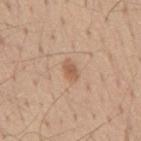Case summary:
- notes · catalogued during a skin exam; not biopsied
- lesion diameter · ~2.5 mm (longest diameter)
- image · ~15 mm crop, total-body skin-cancer survey
- body site · the back
- subject · male, aged 53–57